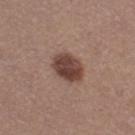Q: Is there a histopathology result?
A: no biopsy performed (imaged during a skin exam)
Q: Illumination type?
A: white-light illumination
Q: Patient demographics?
A: female, aged around 35
Q: What kind of image is this?
A: 15 mm crop, total-body photography
Q: Lesion size?
A: ~4 mm (longest diameter)
Q: Lesion location?
A: the right thigh